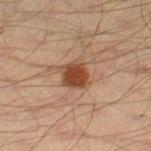Clinical impression:
Part of a total-body skin-imaging series; this lesion was reviewed on a skin check and was not flagged for biopsy.
Context:
The lesion is on the right thigh. Imaged with cross-polarized lighting. A male subject, aged approximately 45. Approximately 3 mm at its widest. A region of skin cropped from a whole-body photographic capture, roughly 15 mm wide. The lesion-visualizer software estimated an area of roughly 7.5 mm², a shape eccentricity near 0.45, and a shape-asymmetry score of about 0.2 (0 = symmetric). The analysis additionally found an average lesion color of about L≈37 a*≈19 b*≈27 (CIELAB), a lesion–skin lightness drop of about 11, and a normalized lesion–skin contrast near 10. The analysis additionally found a border-irregularity index near 2.5/10, a color-variation rating of about 3.5/10, and a peripheral color-asymmetry measure near 1.